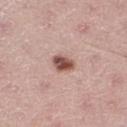A 15 mm close-up extracted from a 3D total-body photography capture. A male patient, aged around 60. From the right thigh.